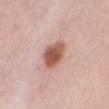  biopsy_status: not biopsied; imaged during a skin examination
  automated_metrics:
    nevus_likeness_0_100: 95
    lesion_detection_confidence_0_100: 100
  patient:
    sex: female
    age_approx: 65
  lighting: white-light
  site: lower back
  image:
    source: total-body photography crop
    field_of_view_mm: 15